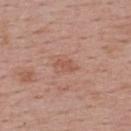{"lighting": "white-light", "lesion_size": {"long_diameter_mm_approx": 2.5}, "image": {"source": "total-body photography crop", "field_of_view_mm": 15}, "site": "upper back", "patient": {"sex": "female", "age_approx": 55}}